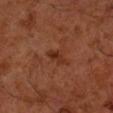Context: From the right forearm. This is a cross-polarized tile. A 15 mm close-up extracted from a 3D total-body photography capture. The patient is a male about 60 years old.